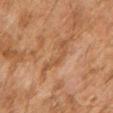Q: Is there a histopathology result?
A: imaged on a skin check; not biopsied
Q: Lesion location?
A: the right upper arm
Q: How was the tile lit?
A: cross-polarized
Q: Patient demographics?
A: female, aged approximately 60
Q: What kind of image is this?
A: ~15 mm crop, total-body skin-cancer survey